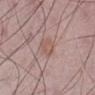Assessment:
The lesion was tiled from a total-body skin photograph and was not biopsied.
Background:
Automated tile analysis of the lesion measured an area of roughly 5 mm², an outline eccentricity of about 0.6 (0 = round, 1 = elongated), and two-axis asymmetry of about 0.2. The analysis additionally found border irregularity of about 1.5 on a 0–10 scale, internal color variation of about 2 on a 0–10 scale, and peripheral color asymmetry of about 1. The analysis additionally found a nevus-likeness score of about 55/100. The recorded lesion diameter is about 3 mm. A male patient, aged 48–52. On the left lower leg. A 15 mm crop from a total-body photograph taken for skin-cancer surveillance.